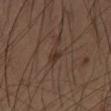notes=catalogued during a skin exam; not biopsied
image source=~15 mm crop, total-body skin-cancer survey
subject=male, aged 58–62
location=the left thigh